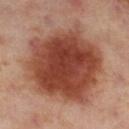Clinical impression:
Part of a total-body skin-imaging series; this lesion was reviewed on a skin check and was not flagged for biopsy.
Acquisition and patient details:
The subject is a female about 55 years old. This image is a 15 mm lesion crop taken from a total-body photograph. On the leg.